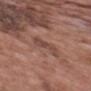Q: Is there a histopathology result?
A: catalogued during a skin exam; not biopsied
Q: What is the imaging modality?
A: ~15 mm tile from a whole-body skin photo
Q: Where on the body is the lesion?
A: the back
Q: Who is the patient?
A: male, approximately 70 years of age